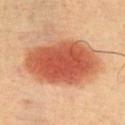Clinical impression: Part of a total-body skin-imaging series; this lesion was reviewed on a skin check and was not flagged for biopsy. Context: Captured under cross-polarized illumination. A 15 mm close-up tile from a total-body photography series done for melanoma screening. Located on the chest. The subject is a male aged around 65.